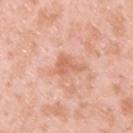Q: Was this lesion biopsied?
A: imaged on a skin check; not biopsied
Q: How large is the lesion?
A: ≈3.5 mm
Q: How was the tile lit?
A: white-light illumination
Q: Lesion location?
A: the back
Q: What is the imaging modality?
A: 15 mm crop, total-body photography
Q: Patient demographics?
A: male, in their mid- to late 30s